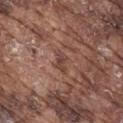The lesion was tiled from a total-body skin photograph and was not biopsied.
An algorithmic analysis of the crop reported a border-irregularity rating of about 4/10, a color-variation rating of about 3/10, and peripheral color asymmetry of about 1. The software also gave an automated nevus-likeness rating near 0 out of 100.
The lesion is located on the mid back.
A 15 mm crop from a total-body photograph taken for skin-cancer surveillance.
The subject is a male aged approximately 75.
Imaged with white-light lighting.